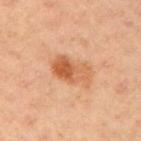Clinical impression: The lesion was tiled from a total-body skin photograph and was not biopsied. Clinical summary: A female patient about 35 years old. Captured under cross-polarized illumination. Located on the arm. The lesion-visualizer software estimated a lesion color around L≈61 a*≈27 b*≈40 in CIELAB, roughly 11 lightness units darker than nearby skin, and a lesion-to-skin contrast of about 8 (normalized; higher = more distinct). It also reported an automated nevus-likeness rating near 70 out of 100 and a detector confidence of about 100 out of 100 that the crop contains a lesion. A region of skin cropped from a whole-body photographic capture, roughly 15 mm wide.